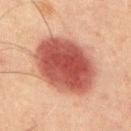<lesion>
<biopsy_status>not biopsied; imaged during a skin examination</biopsy_status>
<image>
  <source>total-body photography crop</source>
  <field_of_view_mm>15</field_of_view_mm>
</image>
<site>chest</site>
<automated_metrics>
  <cielab_L>42</cielab_L>
  <cielab_a>25</cielab_a>
  <cielab_b>25</cielab_b>
  <vs_skin_darker_L>15.0</vs_skin_darker_L>
  <vs_skin_contrast_norm>11.5</vs_skin_contrast_norm>
  <border_irregularity_0_10>1.0</border_irregularity_0_10>
  <peripheral_color_asymmetry>1.5</peripheral_color_asymmetry>
  <nevus_likeness_0_100>100</nevus_likeness_0_100>
  <lesion_detection_confidence_0_100>100</lesion_detection_confidence_0_100>
</automated_metrics>
<patient>
  <sex>male</sex>
  <age_approx>65</age_approx>
</patient>
<lesion_size>
  <long_diameter_mm_approx>8.5</long_diameter_mm_approx>
</lesion_size>
</lesion>